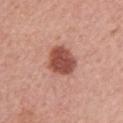Acquisition and patient details:
A 15 mm close-up tile from a total-body photography series done for melanoma screening. The total-body-photography lesion software estimated a mean CIELAB color near L≈50 a*≈27 b*≈28 and roughly 15 lightness units darker than nearby skin. And it measured lesion-presence confidence of about 100/100. The lesion is on the right upper arm. Captured under white-light illumination. A female patient, aged approximately 60.